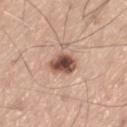Recorded during total-body skin imaging; not selected for excision or biopsy. The lesion is located on the left thigh. A male subject roughly 55 years of age. The total-body-photography lesion software estimated an area of roughly 7.5 mm², a shape eccentricity near 0.3, and a symmetry-axis asymmetry near 0.2. It also reported a lesion color around L≈51 a*≈20 b*≈26 in CIELAB and a normalized lesion–skin contrast near 11.5. The software also gave a border-irregularity index near 2/10, a within-lesion color-variation index near 7/10, and peripheral color asymmetry of about 2. And it measured a classifier nevus-likeness of about 85/100. A 15 mm crop from a total-body photograph taken for skin-cancer surveillance.